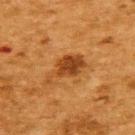Image and clinical context:
A roughly 15 mm field-of-view crop from a total-body skin photograph. A female subject aged approximately 50. Located on the back. The tile uses cross-polarized illumination. Automated tile analysis of the lesion measured an average lesion color of about L≈37 a*≈23 b*≈37 (CIELAB), a lesion–skin lightness drop of about 10, and a normalized border contrast of about 8.5. The software also gave a nevus-likeness score of about 35/100 and a detector confidence of about 100 out of 100 that the crop contains a lesion.